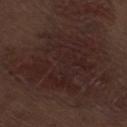<case>
  <biopsy_status>not biopsied; imaged during a skin examination</biopsy_status>
  <automated_metrics>
    <border_irregularity_0_10>7.5</border_irregularity_0_10>
    <color_variation_0_10>3.5</color_variation_0_10>
    <peripheral_color_asymmetry>1.0</peripheral_color_asymmetry>
  </automated_metrics>
  <site>leg</site>
  <lesion_size>
    <long_diameter_mm_approx>11.5</long_diameter_mm_approx>
  </lesion_size>
  <image>
    <source>total-body photography crop</source>
    <field_of_view_mm>15</field_of_view_mm>
  </image>
  <patient>
    <sex>male</sex>
    <age_approx>70</age_approx>
  </patient>
  <lighting>white-light</lighting>
</case>